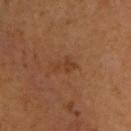notes=no biopsy performed (imaged during a skin exam) | size=≈3 mm | site=the upper back | subject=male, roughly 65 years of age | tile lighting=cross-polarized | imaging modality=total-body-photography crop, ~15 mm field of view.